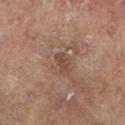notes: no biopsy performed (imaged during a skin exam) | automated metrics: a footprint of about 3.5 mm² and an outline eccentricity of about 0.8 (0 = round, 1 = elongated); an average lesion color of about L≈46 a*≈18 b*≈27 (CIELAB) and a lesion-to-skin contrast of about 6 (normalized; higher = more distinct); a nevus-likeness score of about 0/100 and a detector confidence of about 100 out of 100 that the crop contains a lesion | subject: female, aged 73 to 77 | diameter: about 2.5 mm | acquisition: 15 mm crop, total-body photography | anatomic site: the left lower leg | lighting: cross-polarized illumination.